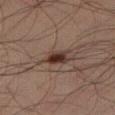{
  "biopsy_status": "not biopsied; imaged during a skin examination",
  "image": {
    "source": "total-body photography crop",
    "field_of_view_mm": 15
  },
  "site": "right thigh",
  "patient": {
    "sex": "male",
    "age_approx": 35
  }
}